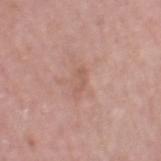The lesion was tiled from a total-body skin photograph and was not biopsied. Automated tile analysis of the lesion measured a shape eccentricity near 0.9. The software also gave an average lesion color of about L≈57 a*≈21 b*≈27 (CIELAB), about 7 CIELAB-L* units darker than the surrounding skin, and a normalized lesion–skin contrast near 5. Cropped from a whole-body photographic skin survey; the tile spans about 15 mm. The recorded lesion diameter is about 2.5 mm. This is a white-light tile. The lesion is on the right thigh. A female subject in their mid- to late 60s.